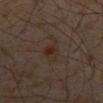The lesion was photographed on a routine skin check and not biopsied; there is no pathology result.
A 15 mm close-up tile from a total-body photography series done for melanoma screening.
The total-body-photography lesion software estimated an average lesion color of about L≈23 a*≈12 b*≈19 (CIELAB) and a normalized border contrast of about 6.5. And it measured border irregularity of about 2.5 on a 0–10 scale, a within-lesion color-variation index near 4.5/10, and peripheral color asymmetry of about 1.5. It also reported a detector confidence of about 100 out of 100 that the crop contains a lesion.
The tile uses cross-polarized illumination.
From the abdomen.
Measured at roughly 2.5 mm in maximum diameter.
A male patient, roughly 60 years of age.